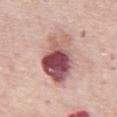lighting: white-light | anatomic site: the abdomen | image: total-body-photography crop, ~15 mm field of view | size: about 7.5 mm | subject: male, in their mid- to late 70s | image-analysis metrics: a mean CIELAB color near L≈54 a*≈25 b*≈21, roughly 19 lightness units darker than nearby skin, and a normalized border contrast of about 12; a border-irregularity index near 3/10, internal color variation of about 10 on a 0–10 scale, and radial color variation of about 4.5.